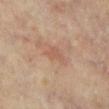Captured during whole-body skin photography for melanoma surveillance; the lesion was not biopsied. A female patient aged 63 to 67. Cropped from a total-body skin-imaging series; the visible field is about 15 mm. On the right leg. Approximately 3 mm at its widest. Imaged with cross-polarized lighting.